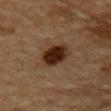Image and clinical context: A region of skin cropped from a whole-body photographic capture, roughly 15 mm wide. From the mid back. A male subject, aged approximately 85 or older.